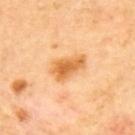Clinical impression: The lesion was photographed on a routine skin check and not biopsied; there is no pathology result. Clinical summary: On the upper back. The subject is a male about 65 years old. A 15 mm crop from a total-body photograph taken for skin-cancer surveillance.